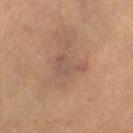<case>
  <biopsy_status>not biopsied; imaged during a skin examination</biopsy_status>
  <image>
    <source>total-body photography crop</source>
    <field_of_view_mm>15</field_of_view_mm>
  </image>
  <patient>
    <sex>female</sex>
    <age_approx>30</age_approx>
  </patient>
  <site>left thigh</site>
  <lighting>cross-polarized</lighting>
  <automated_metrics>
    <border_irregularity_0_10>5.5</border_irregularity_0_10>
    <color_variation_0_10>2.5</color_variation_0_10>
    <peripheral_color_asymmetry>1.0</peripheral_color_asymmetry>
    <nevus_likeness_0_100>0</nevus_likeness_0_100>
  </automated_metrics>
</case>